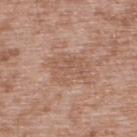Part of a total-body skin-imaging series; this lesion was reviewed on a skin check and was not flagged for biopsy. This is a white-light tile. Measured at roughly 5 mm in maximum diameter. A roughly 15 mm field-of-view crop from a total-body skin photograph. The total-body-photography lesion software estimated an outline eccentricity of about 0.75 (0 = round, 1 = elongated) and a shape-asymmetry score of about 0.3 (0 = symmetric). And it measured a mean CIELAB color near L≈56 a*≈20 b*≈29 and a normalized border contrast of about 4.5. The lesion is on the upper back. The subject is a male aged 48 to 52.